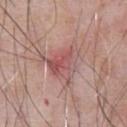The tile uses white-light illumination.
A roughly 15 mm field-of-view crop from a total-body skin photograph.
From the abdomen.
The lesion's longest dimension is about 4.5 mm.
A male patient aged around 60.